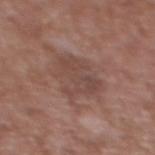  biopsy_status: not biopsied; imaged during a skin examination
  image:
    source: total-body photography crop
    field_of_view_mm: 15
  lesion_size:
    long_diameter_mm_approx: 4.5
  patient:
    sex: male
    age_approx: 45
  site: back